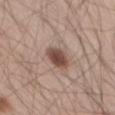Background:
A 15 mm close-up extracted from a 3D total-body photography capture. About 3.5 mm across. A male subject approximately 60 years of age. Automated tile analysis of the lesion measured a border-irregularity index near 1.5/10, a within-lesion color-variation index near 3.5/10, and radial color variation of about 1. It also reported an automated nevus-likeness rating near 95 out of 100. From the leg. Captured under white-light illumination.